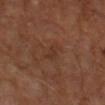Clinical impression:
Captured during whole-body skin photography for melanoma surveillance; the lesion was not biopsied.
Acquisition and patient details:
A lesion tile, about 15 mm wide, cut from a 3D total-body photograph. The subject is a male in their mid-60s. Longest diameter approximately 3 mm. Captured under cross-polarized illumination. The lesion-visualizer software estimated a lesion-to-skin contrast of about 5 (normalized; higher = more distinct). It also reported border irregularity of about 5 on a 0–10 scale, internal color variation of about 0.5 on a 0–10 scale, and a peripheral color-asymmetry measure near 0. It also reported an automated nevus-likeness rating near 0 out of 100. From the left forearm.